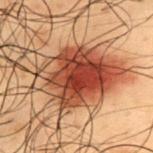Clinical impression:
Part of a total-body skin-imaging series; this lesion was reviewed on a skin check and was not flagged for biopsy.
Image and clinical context:
A 15 mm crop from a total-body photograph taken for skin-cancer surveillance. Longest diameter approximately 8.5 mm. On the upper back. A male patient about 50 years old. Imaged with cross-polarized lighting.